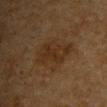biopsy status: no biopsy performed (imaged during a skin exam); lighting: cross-polarized; lesion diameter: ≈6 mm; subject: male, approximately 85 years of age or older; image: ~15 mm crop, total-body skin-cancer survey; site: the chest.